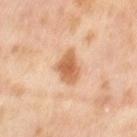biopsy_status: not biopsied; imaged during a skin examination
patient:
  sex: female
  age_approx: 50
site: left thigh
image:
  source: total-body photography crop
  field_of_view_mm: 15
lesion_size:
  long_diameter_mm_approx: 4.5
automated_metrics:
  area_mm2_approx: 8.5
  eccentricity: 0.85
  shape_asymmetry: 0.25
  vs_skin_darker_L: 13.0
  vs_skin_contrast_norm: 8.5
  border_irregularity_0_10: 2.5
  color_variation_0_10: 3.0
  peripheral_color_asymmetry: 0.5
  nevus_likeness_0_100: 75
lighting: cross-polarized